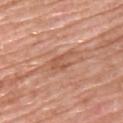{
  "biopsy_status": "not biopsied; imaged during a skin examination",
  "patient": {
    "sex": "male",
    "age_approx": 50
  },
  "lesion_size": {
    "long_diameter_mm_approx": 3.5
  },
  "site": "upper back",
  "image": {
    "source": "total-body photography crop",
    "field_of_view_mm": 15
  },
  "automated_metrics": {
    "area_mm2_approx": 5.0,
    "eccentricity": 0.8,
    "shape_asymmetry": 0.4,
    "border_irregularity_0_10": 4.5,
    "color_variation_0_10": 2.0,
    "lesion_detection_confidence_0_100": 90
  }
}